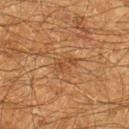<case>
  <lighting>cross-polarized</lighting>
  <site>right lower leg</site>
  <patient>
    <sex>male</sex>
    <age_approx>60</age_approx>
  </patient>
  <image>
    <source>total-body photography crop</source>
    <field_of_view_mm>15</field_of_view_mm>
  </image>
</case>